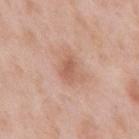The lesion was tiled from a total-body skin photograph and was not biopsied. Measured at roughly 3 mm in maximum diameter. A male subject, roughly 55 years of age. Automated tile analysis of the lesion measured an average lesion color of about L≈59 a*≈23 b*≈30 (CIELAB) and a normalized lesion–skin contrast near 6. This image is a 15 mm lesion crop taken from a total-body photograph. The lesion is located on the mid back.